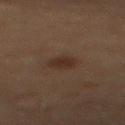Part of a total-body skin-imaging series; this lesion was reviewed on a skin check and was not flagged for biopsy.
A lesion tile, about 15 mm wide, cut from a 3D total-body photograph.
From the mid back.
This is a cross-polarized tile.
A male subject roughly 70 years of age.
An algorithmic analysis of the crop reported a footprint of about 5 mm² and a shape-asymmetry score of about 0.2 (0 = symmetric). And it measured a border-irregularity rating of about 2/10, a color-variation rating of about 1/10, and peripheral color asymmetry of about 0.5.
The lesion's longest dimension is about 3 mm.